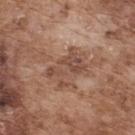A male patient, about 75 years old. Automated tile analysis of the lesion measured an outline eccentricity of about 0.8 (0 = round, 1 = elongated). The software also gave a nevus-likeness score of about 0/100 and lesion-presence confidence of about 100/100. From the back. The recorded lesion diameter is about 5 mm. Cropped from a whole-body photographic skin survey; the tile spans about 15 mm.